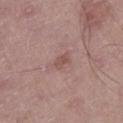A male patient in their mid- to late 60s.
Located on the left lower leg.
A lesion tile, about 15 mm wide, cut from a 3D total-body photograph.